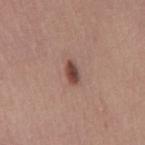Clinical impression:
Part of a total-body skin-imaging series; this lesion was reviewed on a skin check and was not flagged for biopsy.
Background:
Approximately 3 mm at its widest. The patient is a female approximately 50 years of age. The lesion is on the mid back. A roughly 15 mm field-of-view crop from a total-body skin photograph. This is a white-light tile.